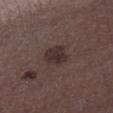No biopsy was performed on this lesion — it was imaged during a full skin examination and was not determined to be concerning. The lesion-visualizer software estimated an area of roughly 7.5 mm². The software also gave roughly 8 lightness units darker than nearby skin. Cropped from a total-body skin-imaging series; the visible field is about 15 mm. The recorded lesion diameter is about 3.5 mm. The patient is a male aged approximately 55. Captured under white-light illumination. The lesion is located on the leg.